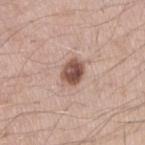The lesion was tiled from a total-body skin photograph and was not biopsied. A male subject, approximately 25 years of age. A roughly 15 mm field-of-view crop from a total-body skin photograph. The recorded lesion diameter is about 3 mm. The lesion is on the right upper arm. Automated image analysis of the tile measured an area of roughly 6.5 mm², an eccentricity of roughly 0.65, and a symmetry-axis asymmetry near 0.15. And it measured a mean CIELAB color near L≈52 a*≈20 b*≈25, about 16 CIELAB-L* units darker than the surrounding skin, and a normalized border contrast of about 10.5. Captured under white-light illumination.